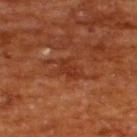Impression: Captured during whole-body skin photography for melanoma surveillance; the lesion was not biopsied. Acquisition and patient details: On the upper back. A lesion tile, about 15 mm wide, cut from a 3D total-body photograph. A male subject, aged 63 to 67.